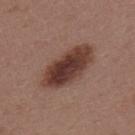Imaged during a routine full-body skin examination; the lesion was not biopsied and no histopathology is available. The subject is a female aged approximately 45. Cropped from a total-body skin-imaging series; the visible field is about 15 mm. Automated tile analysis of the lesion measured a border-irregularity rating of about 2.5/10 and a color-variation rating of about 5.5/10. The software also gave a classifier nevus-likeness of about 20/100 and a detector confidence of about 100 out of 100 that the crop contains a lesion. On the upper back. Imaged with white-light lighting. Approximately 7 mm at its widest.